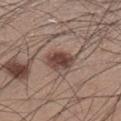Findings:
– biopsy status: imaged on a skin check; not biopsied
– anatomic site: the left lower leg
– image source: total-body-photography crop, ~15 mm field of view
– patient: male, about 35 years old